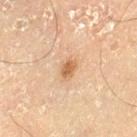Clinical summary:
The patient is a male roughly 70 years of age. Captured under cross-polarized illumination. On the left thigh. A close-up tile cropped from a whole-body skin photograph, about 15 mm across. Measured at roughly 2.5 mm in maximum diameter.